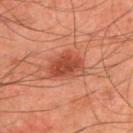The lesion was photographed on a routine skin check and not biopsied; there is no pathology result. This image is a 15 mm lesion crop taken from a total-body photograph. On the back. A male patient aged 43–47. About 4.5 mm across.